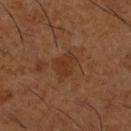No biopsy was performed on this lesion — it was imaged during a full skin examination and was not determined to be concerning. A close-up tile cropped from a whole-body skin photograph, about 15 mm across. A male subject aged around 65. From the right lower leg.